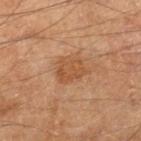biopsy status: catalogued during a skin exam; not biopsied
site: the leg
TBP lesion metrics: an area of roughly 7.5 mm² and an eccentricity of roughly 0.6
image: ~15 mm tile from a whole-body skin photo
size: ~3.5 mm (longest diameter)
subject: male, in their mid- to late 60s
lighting: cross-polarized illumination